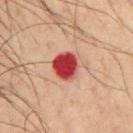Located on the chest. A male subject, about 45 years old. A 15 mm crop from a total-body photograph taken for skin-cancer surveillance.